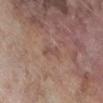Clinical impression: Recorded during total-body skin imaging; not selected for excision or biopsy.